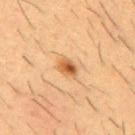Imaged during a routine full-body skin examination; the lesion was not biopsied and no histopathology is available.